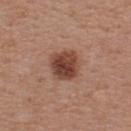This lesion was catalogued during total-body skin photography and was not selected for biopsy.
The tile uses white-light illumination.
A male subject aged around 55.
Measured at roughly 3.5 mm in maximum diameter.
Cropped from a total-body skin-imaging series; the visible field is about 15 mm.
Located on the back.